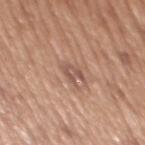Notes:
* notes · total-body-photography surveillance lesion; no biopsy
* size · ~2.5 mm (longest diameter)
* subject · male, aged around 65
* site · the mid back
* image source · ~15 mm crop, total-body skin-cancer survey
* image-analysis metrics · an area of roughly 3.5 mm², an outline eccentricity of about 0.7 (0 = round, 1 = elongated), and a symmetry-axis asymmetry near 0.35; a mean CIELAB color near L≈54 a*≈20 b*≈26, roughly 9 lightness units darker than nearby skin, and a lesion-to-skin contrast of about 6 (normalized; higher = more distinct); a border-irregularity rating of about 4/10 and a peripheral color-asymmetry measure near 0.5